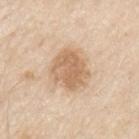follow-up = no biopsy performed (imaged during a skin exam)
site = the chest
automated metrics = a border-irregularity rating of about 2.5/10, a color-variation rating of about 3.5/10, and radial color variation of about 1; a classifier nevus-likeness of about 10/100 and a lesion-detection confidence of about 100/100
lesion diameter = about 4.5 mm
acquisition = 15 mm crop, total-body photography
patient = male, approximately 80 years of age